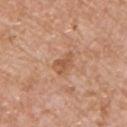  biopsy_status: not biopsied; imaged during a skin examination
  lesion_size:
    long_diameter_mm_approx: 3.0
  lighting: white-light
  patient:
    sex: male
    age_approx: 70
  site: chest
  image:
    source: total-body photography crop
    field_of_view_mm: 15